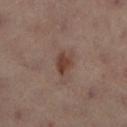{
  "biopsy_status": "not biopsied; imaged during a skin examination",
  "image": {
    "source": "total-body photography crop",
    "field_of_view_mm": 15
  },
  "site": "left lower leg",
  "lighting": "cross-polarized",
  "patient": {
    "sex": "female",
    "age_approx": 55
  },
  "automated_metrics": {
    "cielab_L": 31,
    "cielab_a": 16,
    "cielab_b": 21,
    "vs_skin_darker_L": 9.0,
    "vs_skin_contrast_norm": 9.0,
    "nevus_likeness_0_100": 95,
    "lesion_detection_confidence_0_100": 100
  }
}